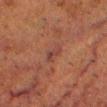Automated tile analysis of the lesion measured a mean CIELAB color near L≈33 a*≈20 b*≈21, roughly 5 lightness units darker than nearby skin, and a lesion-to-skin contrast of about 6 (normalized; higher = more distinct). The software also gave border irregularity of about 3.5 on a 0–10 scale, internal color variation of about 3.5 on a 0–10 scale, and radial color variation of about 1.5. The software also gave a lesion-detection confidence of about 95/100. The recorded lesion diameter is about 2.5 mm. A region of skin cropped from a whole-body photographic capture, roughly 15 mm wide. The patient is a male about 50 years old. On the head or neck.